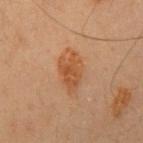Impression: No biopsy was performed on this lesion — it was imaged during a full skin examination and was not determined to be concerning. Clinical summary: A region of skin cropped from a whole-body photographic capture, roughly 15 mm wide. The recorded lesion diameter is about 5 mm. A male patient, about 50 years old. Located on the chest.